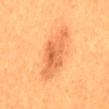Part of a total-body skin-imaging series; this lesion was reviewed on a skin check and was not flagged for biopsy. The lesion's longest dimension is about 7.5 mm. The subject is a female in their 30s. The lesion-visualizer software estimated an area of roughly 15 mm², an eccentricity of roughly 0.95, and a shape-asymmetry score of about 0.25 (0 = symmetric). The software also gave an average lesion color of about L≈61 a*≈29 b*≈43 (CIELAB), a lesion–skin lightness drop of about 11, and a lesion-to-skin contrast of about 6.5 (normalized; higher = more distinct). It also reported a border-irregularity rating of about 4.5/10, a color-variation rating of about 4.5/10, and radial color variation of about 1.5. The tile uses cross-polarized illumination. Located on the mid back. A region of skin cropped from a whole-body photographic capture, roughly 15 mm wide.